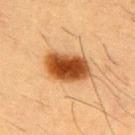image source: ~15 mm tile from a whole-body skin photo | body site: the chest | patient: male, about 55 years old.